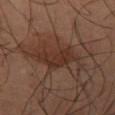Captured during whole-body skin photography for melanoma surveillance; the lesion was not biopsied. A region of skin cropped from a whole-body photographic capture, roughly 15 mm wide. A male subject, in their mid- to late 60s. The lesion is on the right thigh. The recorded lesion diameter is about 5 mm.